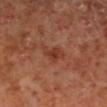Imaged during a routine full-body skin examination; the lesion was not biopsied and no histopathology is available. The recorded lesion diameter is about 3.5 mm. The lesion is located on the right lower leg. A lesion tile, about 15 mm wide, cut from a 3D total-body photograph. Imaged with cross-polarized lighting. The patient is a male roughly 60 years of age.